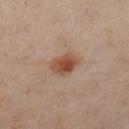Assessment:
The lesion was tiled from a total-body skin photograph and was not biopsied.
Image and clinical context:
The patient is a female aged approximately 40. A roughly 15 mm field-of-view crop from a total-body skin photograph. The lesion is located on the right leg.